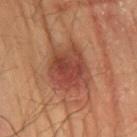Located on the lower back. The patient is a male about 50 years old. A lesion tile, about 15 mm wide, cut from a 3D total-body photograph.